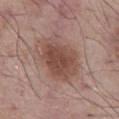  biopsy_status: not biopsied; imaged during a skin examination
  image:
    source: total-body photography crop
    field_of_view_mm: 15
  site: abdomen
  patient:
    sex: male
    age_approx: 55
  automated_metrics:
    cielab_L: 48
    cielab_a: 20
    cielab_b: 24
    vs_skin_darker_L: 10.0
    vs_skin_contrast_norm: 8.0